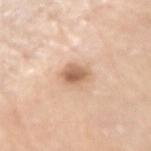Assessment:
Part of a total-body skin-imaging series; this lesion was reviewed on a skin check and was not flagged for biopsy.
Context:
A male patient, roughly 80 years of age. Imaged with white-light lighting. A lesion tile, about 15 mm wide, cut from a 3D total-body photograph. Located on the arm. About 3 mm across.